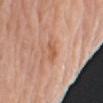This lesion was catalogued during total-body skin photography and was not selected for biopsy.
About 2.5 mm across.
A male subject, about 80 years old.
The lesion is located on the right upper arm.
A region of skin cropped from a whole-body photographic capture, roughly 15 mm wide.
The tile uses white-light illumination.